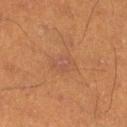| key | value |
|---|---|
| tile lighting | cross-polarized |
| lesion size | ~3.5 mm (longest diameter) |
| image source | 15 mm crop, total-body photography |
| site | the left lower leg |
| subject | male, aged 38 to 42 |
| TBP lesion metrics | a lesion color around L≈42 a*≈19 b*≈27 in CIELAB and about 4 CIELAB-L* units darker than the surrounding skin |